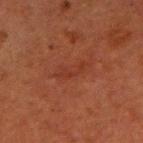biopsy_status: not biopsied; imaged during a skin examination
lighting: cross-polarized
image:
  source: total-body photography crop
  field_of_view_mm: 15
lesion_size:
  long_diameter_mm_approx: 3.5
patient:
  sex: male
  age_approx: 65
site: left upper arm